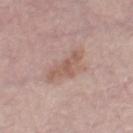{"biopsy_status": "not biopsied; imaged during a skin examination", "patient": {"sex": "female", "age_approx": 70}, "image": {"source": "total-body photography crop", "field_of_view_mm": 15}, "lesion_size": {"long_diameter_mm_approx": 4.5}, "site": "right thigh"}